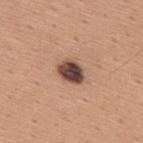* notes — no biopsy performed (imaged during a skin exam)
* size — ≈3.5 mm
* illumination — white-light
* automated lesion analysis — a footprint of about 6.5 mm², an eccentricity of roughly 0.7, and a symmetry-axis asymmetry near 0.2
* site — the upper back
* subject — male, aged approximately 45
* image source — ~15 mm tile from a whole-body skin photo